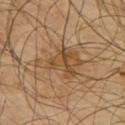follow-up: imaged on a skin check; not biopsied | diameter: ≈6 mm | subject: male, aged 63 to 67 | site: the upper back | image: ~15 mm crop, total-body skin-cancer survey | tile lighting: cross-polarized illumination | TBP lesion metrics: a lesion color around L≈46 a*≈16 b*≈34 in CIELAB and a normalized border contrast of about 6.5; a border-irregularity index near 6/10, a color-variation rating of about 5.5/10, and radial color variation of about 2.